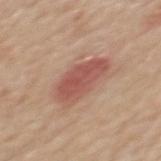Imaged during a routine full-body skin examination; the lesion was not biopsied and no histopathology is available.
A female subject roughly 50 years of age.
A roughly 15 mm field-of-view crop from a total-body skin photograph.
This is a white-light tile.
Located on the upper back.